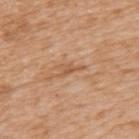| key | value |
|---|---|
| biopsy status | total-body-photography surveillance lesion; no biopsy |
| tile lighting | white-light illumination |
| TBP lesion metrics | a lesion area of about 3.5 mm² and a symmetry-axis asymmetry near 0.5; border irregularity of about 6 on a 0–10 scale and radial color variation of about 0.5; a nevus-likeness score of about 0/100 and a lesion-detection confidence of about 60/100 |
| patient | female, roughly 40 years of age |
| site | the left upper arm |
| imaging modality | total-body-photography crop, ~15 mm field of view |
| lesion diameter | ≈3.5 mm |